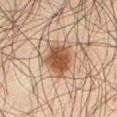No biopsy was performed on this lesion — it was imaged during a full skin examination and was not determined to be concerning. A male subject aged 63–67. The tile uses cross-polarized illumination. This image is a 15 mm lesion crop taken from a total-body photograph. An algorithmic analysis of the crop reported a footprint of about 14 mm², an eccentricity of roughly 0.65, and a symmetry-axis asymmetry near 0.3. The software also gave border irregularity of about 3.5 on a 0–10 scale, a within-lesion color-variation index near 4/10, and radial color variation of about 1. The software also gave a classifier nevus-likeness of about 100/100 and a lesion-detection confidence of about 100/100. The lesion is on the front of the torso. Measured at roughly 5 mm in maximum diameter.